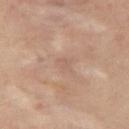This lesion was catalogued during total-body skin photography and was not selected for biopsy. A male subject, aged around 40. Captured under cross-polarized illumination. Longest diameter approximately 2 mm. From the abdomen. A lesion tile, about 15 mm wide, cut from a 3D total-body photograph.